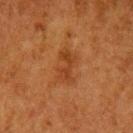The lesion's longest dimension is about 4 mm. A female subject aged 48 to 52. A lesion tile, about 15 mm wide, cut from a 3D total-body photograph. The lesion is located on the arm.